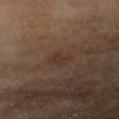Recorded during total-body skin imaging; not selected for excision or biopsy. Located on the right upper arm. Imaged with cross-polarized lighting. The total-body-photography lesion software estimated an eccentricity of roughly 0.7 and two-axis asymmetry of about 0.4. It also reported a border-irregularity index near 4/10, a within-lesion color-variation index near 1/10, and peripheral color asymmetry of about 0.5. The software also gave a classifier nevus-likeness of about 0/100 and a detector confidence of about 100 out of 100 that the crop contains a lesion. The subject is a female aged around 80. A 15 mm crop from a total-body photograph taken for skin-cancer surveillance. Approximately 2.5 mm at its widest.